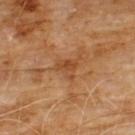follow-up — no biopsy performed (imaged during a skin exam) | location — the chest | image-analysis metrics — an outline eccentricity of about 0.6 (0 = round, 1 = elongated) and a shape-asymmetry score of about 0.45 (0 = symmetric); a color-variation rating of about 2.5/10 and a peripheral color-asymmetry measure near 1; a nevus-likeness score of about 0/100 and lesion-presence confidence of about 100/100 | image — ~15 mm tile from a whole-body skin photo | diameter — about 2.5 mm | subject — male, in their 60s | illumination — cross-polarized.